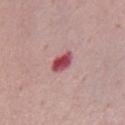workup = catalogued during a skin exam; not biopsied | lesion size = about 3.5 mm | acquisition = 15 mm crop, total-body photography | tile lighting = white-light illumination | subject = female, roughly 50 years of age | anatomic site = the front of the torso.